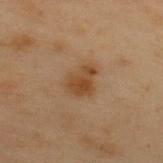Findings:
* follow-up: total-body-photography surveillance lesion; no biopsy
* site: the back
* subject: male, in their mid- to late 50s
* acquisition: total-body-photography crop, ~15 mm field of view